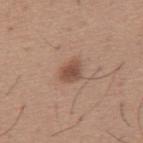The lesion was photographed on a routine skin check and not biopsied; there is no pathology result. From the mid back. The subject is a male aged 63 to 67. This image is a 15 mm lesion crop taken from a total-body photograph.